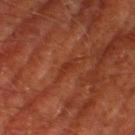Clinical impression: The lesion was tiled from a total-body skin photograph and was not biopsied. Acquisition and patient details: The patient is a male approximately 65 years of age. An algorithmic analysis of the crop reported a lesion color around L≈33 a*≈29 b*≈32 in CIELAB, a lesion–skin lightness drop of about 6, and a lesion-to-skin contrast of about 5.5 (normalized; higher = more distinct). From the right thigh. About 2.5 mm across. The tile uses cross-polarized illumination. A lesion tile, about 15 mm wide, cut from a 3D total-body photograph.